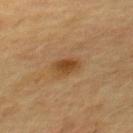This is a cross-polarized tile.
Located on the upper back.
About 3 mm across.
Cropped from a whole-body photographic skin survey; the tile spans about 15 mm.
A female subject, aged approximately 60.